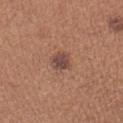Q: Was this lesion biopsied?
A: imaged on a skin check; not biopsied
Q: Who is the patient?
A: female, about 55 years old
Q: How was this image acquired?
A: ~15 mm crop, total-body skin-cancer survey
Q: What is the lesion's diameter?
A: ≈2.5 mm
Q: Illumination type?
A: white-light
Q: Lesion location?
A: the left upper arm
Q: What did automated image analysis measure?
A: a lesion area of about 4.5 mm² and a shape-asymmetry score of about 0.2 (0 = symmetric); a mean CIELAB color near L≈46 a*≈19 b*≈23 and a normalized lesion–skin contrast near 9; peripheral color asymmetry of about 1; a classifier nevus-likeness of about 80/100 and a lesion-detection confidence of about 100/100